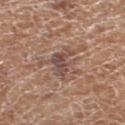Recorded during total-body skin imaging; not selected for excision or biopsy.
Captured under white-light illumination.
This image is a 15 mm lesion crop taken from a total-body photograph.
The lesion is on the left thigh.
Longest diameter approximately 4.5 mm.
A female patient aged 73 to 77.